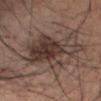Q: Was this lesion biopsied?
A: total-body-photography surveillance lesion; no biopsy